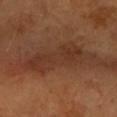follow-up: total-body-photography surveillance lesion; no biopsy
illumination: cross-polarized
subject: female, aged approximately 70
TBP lesion metrics: a lesion area of about 18 mm², a shape eccentricity near 0.9, and two-axis asymmetry of about 0.35; a lesion-to-skin contrast of about 6.5 (normalized; higher = more distinct); a border-irregularity index near 5.5/10, a within-lesion color-variation index near 3/10, and peripheral color asymmetry of about 1
image source: 15 mm crop, total-body photography
body site: the left forearm